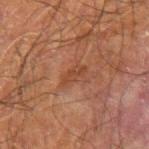<case>
  <biopsy_status>not biopsied; imaged during a skin examination</biopsy_status>
  <site>right forearm</site>
  <image>
    <source>total-body photography crop</source>
    <field_of_view_mm>15</field_of_view_mm>
  </image>
  <patient>
    <sex>male</sex>
    <age_approx>70</age_approx>
  </patient>
  <lighting>cross-polarized</lighting>
  <lesion_size>
    <long_diameter_mm_approx>3.0</long_diameter_mm_approx>
  </lesion_size>
</case>